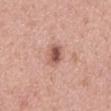| field | value |
|---|---|
| notes | total-body-photography surveillance lesion; no biopsy |
| location | the mid back |
| patient | male, in their 40s |
| lesion size | about 3.5 mm |
| acquisition | ~15 mm tile from a whole-body skin photo |